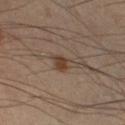Imaged during a routine full-body skin examination; the lesion was not biopsied and no histopathology is available.
A male patient, aged approximately 55.
A lesion tile, about 15 mm wide, cut from a 3D total-body photograph.
An algorithmic analysis of the crop reported a footprint of about 5 mm², an outline eccentricity of about 0.8 (0 = round, 1 = elongated), and a shape-asymmetry score of about 0.35 (0 = symmetric). The software also gave a classifier nevus-likeness of about 90/100 and a lesion-detection confidence of about 100/100.
The lesion is located on the right lower leg.
The tile uses cross-polarized illumination.
Approximately 3.5 mm at its widest.